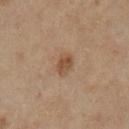| field | value |
|---|---|
| workup | no biopsy performed (imaged during a skin exam) |
| TBP lesion metrics | a lesion color around L≈51 a*≈19 b*≈32 in CIELAB and a lesion–skin lightness drop of about 9; a border-irregularity index near 2/10 and peripheral color asymmetry of about 1.5; a nevus-likeness score of about 70/100 |
| anatomic site | the left lower leg |
| lighting | cross-polarized illumination |
| subject | female, in their 70s |
| acquisition | total-body-photography crop, ~15 mm field of view |
| size | about 3 mm |